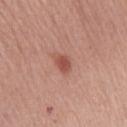Imaged during a routine full-body skin examination; the lesion was not biopsied and no histopathology is available. On the left forearm. A roughly 15 mm field-of-view crop from a total-body skin photograph. A female patient in their mid- to late 60s.